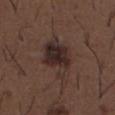Assessment: Captured during whole-body skin photography for melanoma surveillance; the lesion was not biopsied. Image and clinical context: A male patient, aged around 50. A 15 mm crop from a total-body photograph taken for skin-cancer surveillance. This is a white-light tile. The lesion is on the abdomen.